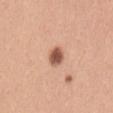Q: Was this lesion biopsied?
A: no biopsy performed (imaged during a skin exam)
Q: Lesion location?
A: the front of the torso
Q: Illumination type?
A: white-light
Q: How was this image acquired?
A: ~15 mm tile from a whole-body skin photo
Q: How large is the lesion?
A: ~2.5 mm (longest diameter)
Q: Who is the patient?
A: female, aged 28 to 32